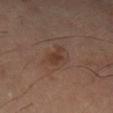This lesion was catalogued during total-body skin photography and was not selected for biopsy.